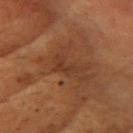Recorded during total-body skin imaging; not selected for excision or biopsy.
The tile uses cross-polarized illumination.
A female patient, approximately 80 years of age.
Longest diameter approximately 3.5 mm.
A close-up tile cropped from a whole-body skin photograph, about 15 mm across.
From the head or neck.